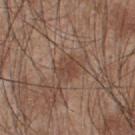workup = total-body-photography surveillance lesion; no biopsy
subject = male, about 45 years old
site = the upper back
image = 15 mm crop, total-body photography
lighting = white-light
lesion size = about 3.5 mm